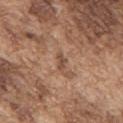Assessment: The lesion was photographed on a routine skin check and not biopsied; there is no pathology result. Context: The lesion is located on the upper back. A roughly 15 mm field-of-view crop from a total-body skin photograph. The patient is a male aged around 75.